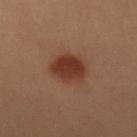Q: Is there a histopathology result?
A: no biopsy performed (imaged during a skin exam)
Q: Where on the body is the lesion?
A: the abdomen
Q: What are the patient's age and sex?
A: female, approximately 30 years of age
Q: How was the tile lit?
A: cross-polarized
Q: How was this image acquired?
A: 15 mm crop, total-body photography